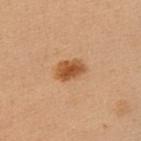Part of a total-body skin-imaging series; this lesion was reviewed on a skin check and was not flagged for biopsy. The lesion is on the chest. Cropped from a whole-body photographic skin survey; the tile spans about 15 mm. The patient is a female aged 48–52. The lesion's longest dimension is about 3.5 mm. The tile uses cross-polarized illumination.